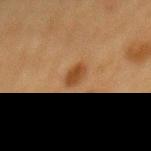{"biopsy_status": "not biopsied; imaged during a skin examination", "image": {"source": "total-body photography crop", "field_of_view_mm": 15}, "patient": {"sex": "female", "age_approx": 55}, "site": "back"}